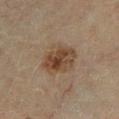Part of a total-body skin-imaging series; this lesion was reviewed on a skin check and was not flagged for biopsy. Automated tile analysis of the lesion measured a shape eccentricity near 0.5 and a shape-asymmetry score of about 0.2 (0 = symmetric). It also reported a nevus-likeness score of about 85/100 and lesion-presence confidence of about 100/100. Captured under cross-polarized illumination. On the left lower leg. Cropped from a total-body skin-imaging series; the visible field is about 15 mm. Longest diameter approximately 5 mm. A male patient roughly 65 years of age.